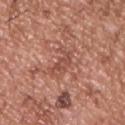This lesion was catalogued during total-body skin photography and was not selected for biopsy. A male subject, in their mid-50s. Captured under white-light illumination. An algorithmic analysis of the crop reported an area of roughly 5.5 mm² and a symmetry-axis asymmetry near 0.5. And it measured border irregularity of about 5.5 on a 0–10 scale, internal color variation of about 2.5 on a 0–10 scale, and a peripheral color-asymmetry measure near 0.5. Approximately 3.5 mm at its widest. The lesion is located on the upper back. Cropped from a whole-body photographic skin survey; the tile spans about 15 mm.